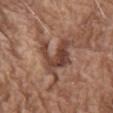The lesion was photographed on a routine skin check and not biopsied; there is no pathology result.
Cropped from a whole-body photographic skin survey; the tile spans about 15 mm.
Measured at roughly 5 mm in maximum diameter.
The patient is a male aged approximately 75.
The lesion is located on the chest.
This is a white-light tile.